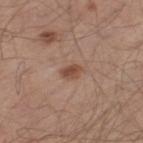Imaged during a routine full-body skin examination; the lesion was not biopsied and no histopathology is available. The subject is a male roughly 65 years of age. On the left thigh. A roughly 15 mm field-of-view crop from a total-body skin photograph.